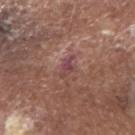workup: catalogued during a skin exam; not biopsied
body site: the head or neck
lesion size: ≈3 mm
illumination: white-light
imaging modality: total-body-photography crop, ~15 mm field of view
subject: male, aged approximately 80